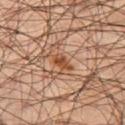The lesion was photographed on a routine skin check and not biopsied; there is no pathology result.